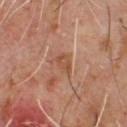Findings:
- notes: imaged on a skin check; not biopsied
- location: the chest
- subject: male, in their 60s
- image source: ~15 mm crop, total-body skin-cancer survey